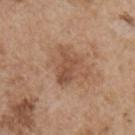{"lesion_size": {"long_diameter_mm_approx": 4.5}, "image": {"source": "total-body photography crop", "field_of_view_mm": 15}, "patient": {"sex": "male", "age_approx": 55}, "lighting": "white-light", "site": "chest"}